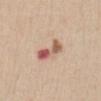This lesion was catalogued during total-body skin photography and was not selected for biopsy. From the front of the torso. The lesion-visualizer software estimated an average lesion color of about L≈58 a*≈23 b*≈28 (CIELAB). It also reported a border-irregularity rating of about 4.5/10 and a peripheral color-asymmetry measure near 7. The software also gave lesion-presence confidence of about 100/100. Approximately 4 mm at its widest. The patient is a female aged approximately 40. A 15 mm close-up tile from a total-body photography series done for melanoma screening.